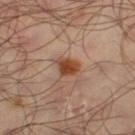Notes:
- workup: imaged on a skin check; not biopsied
- lighting: cross-polarized illumination
- site: the left thigh
- image-analysis metrics: an area of roughly 5 mm², a shape eccentricity near 0.6, and a symmetry-axis asymmetry near 0.3; border irregularity of about 2.5 on a 0–10 scale, a within-lesion color-variation index near 3/10, and a peripheral color-asymmetry measure near 1; a nevus-likeness score of about 100/100
- acquisition: ~15 mm tile from a whole-body skin photo
- subject: male, about 65 years old